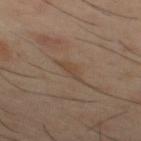follow-up: catalogued during a skin exam; not biopsied
site: the mid back
acquisition: ~15 mm tile from a whole-body skin photo
tile lighting: cross-polarized
automated lesion analysis: a footprint of about 2.5 mm², an eccentricity of roughly 0.9, and a shape-asymmetry score of about 0.4 (0 = symmetric); a lesion color around L≈42 a*≈14 b*≈27 in CIELAB, about 6 CIELAB-L* units darker than the surrounding skin, and a normalized border contrast of about 5.5; a border-irregularity index near 4/10 and internal color variation of about 0 on a 0–10 scale
subject: male, about 40 years old